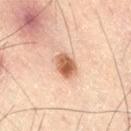Captured during whole-body skin photography for melanoma surveillance; the lesion was not biopsied. About 3.5 mm across. On the right thigh. An algorithmic analysis of the crop reported an area of roughly 6.5 mm², an eccentricity of roughly 0.65, and two-axis asymmetry of about 0.2. And it measured an average lesion color of about L≈55 a*≈21 b*≈31 (CIELAB), roughly 15 lightness units darker than nearby skin, and a normalized border contrast of about 10. This is a cross-polarized tile. A male patient aged around 50. Cropped from a whole-body photographic skin survey; the tile spans about 15 mm.